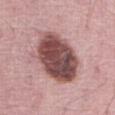anatomic site = the abdomen
imaging modality = ~15 mm tile from a whole-body skin photo
lesion size = ~7.5 mm (longest diameter)
patient = male, about 70 years old
tile lighting = white-light illumination